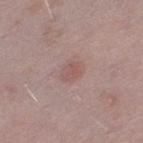Imaged during a routine full-body skin examination; the lesion was not biopsied and no histopathology is available. A 15 mm crop from a total-body photograph taken for skin-cancer surveillance. Approximately 2.5 mm at its widest. Imaged with white-light lighting. Located on the left thigh. The subject is a male roughly 40 years of age.